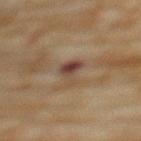Impression: The lesion was tiled from a total-body skin photograph and was not biopsied. Image and clinical context: Longest diameter approximately 3.5 mm. The total-body-photography lesion software estimated a border-irregularity rating of about 5.5/10, internal color variation of about 4.5 on a 0–10 scale, and a peripheral color-asymmetry measure near 1. Imaged with cross-polarized lighting. The patient is a female in their 80s. On the back. A 15 mm close-up tile from a total-body photography series done for melanoma screening.